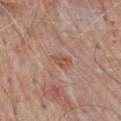The lesion was tiled from a total-body skin photograph and was not biopsied.
A 15 mm crop from a total-body photograph taken for skin-cancer surveillance.
A male patient, in their mid-70s.
The lesion is located on the mid back.
An algorithmic analysis of the crop reported an area of roughly 5 mm², an outline eccentricity of about 0.8 (0 = round, 1 = elongated), and two-axis asymmetry of about 0.2. The software also gave an average lesion color of about L≈54 a*≈19 b*≈28 (CIELAB), about 6 CIELAB-L* units darker than the surrounding skin, and a normalized border contrast of about 5. It also reported lesion-presence confidence of about 100/100.
This is a white-light tile.
Approximately 3 mm at its widest.